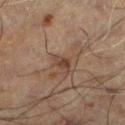{"lesion_size": {"long_diameter_mm_approx": 2.5}, "patient": {"sex": "male", "age_approx": 60}, "lighting": "cross-polarized", "site": "left lower leg", "image": {"source": "total-body photography crop", "field_of_view_mm": 15}}